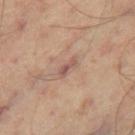Recorded during total-body skin imaging; not selected for excision or biopsy.
A male subject aged around 50.
A region of skin cropped from a whole-body photographic capture, roughly 15 mm wide.
The lesion is on the left leg.